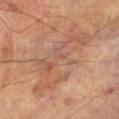No biopsy was performed on this lesion — it was imaged during a full skin examination and was not determined to be concerning. A 15 mm crop from a total-body photograph taken for skin-cancer surveillance. The lesion is on the leg. This is a cross-polarized tile. The subject is a male roughly 70 years of age.